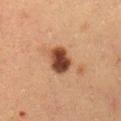patient: female, aged approximately 50 | lighting: cross-polarized | lesion diameter: ~3.5 mm (longest diameter) | location: the abdomen | image source: ~15 mm crop, total-body skin-cancer survey.